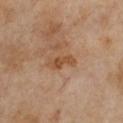workup: no biopsy performed (imaged during a skin exam); diameter: ≈3.5 mm; imaging modality: ~15 mm crop, total-body skin-cancer survey; illumination: cross-polarized illumination; subject: female, aged around 70; body site: the chest.